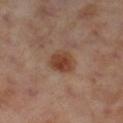Part of a total-body skin-imaging series; this lesion was reviewed on a skin check and was not flagged for biopsy. Cropped from a whole-body photographic skin survey; the tile spans about 15 mm. A female subject, about 55 years old. The lesion's longest dimension is about 3 mm. The lesion is located on the right lower leg.